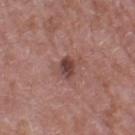<record>
  <biopsy_status>not biopsied; imaged during a skin examination</biopsy_status>
  <patient>
    <sex>male</sex>
    <age_approx>60</age_approx>
  </patient>
  <site>right thigh</site>
  <image>
    <source>total-body photography crop</source>
    <field_of_view_mm>15</field_of_view_mm>
  </image>
  <lesion_size>
    <long_diameter_mm_approx>2.5</long_diameter_mm_approx>
  </lesion_size>
</record>